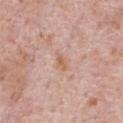Imaged during a routine full-body skin examination; the lesion was not biopsied and no histopathology is available.
The lesion is on the chest.
The subject is a male aged approximately 70.
This is a white-light tile.
Measured at roughly 2.5 mm in maximum diameter.
A 15 mm close-up extracted from a 3D total-body photography capture.